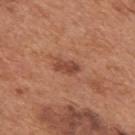Assessment:
Imaged during a routine full-body skin examination; the lesion was not biopsied and no histopathology is available.
Background:
A male subject aged 63 to 67. From the mid back. A roughly 15 mm field-of-view crop from a total-body skin photograph. This is a white-light tile.